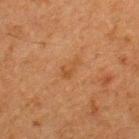Recorded during total-body skin imaging; not selected for excision or biopsy.